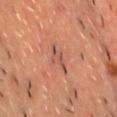| key | value |
|---|---|
| workup | catalogued during a skin exam; not biopsied |
| patient | male, aged approximately 45 |
| body site | the chest |
| image | ~15 mm tile from a whole-body skin photo |